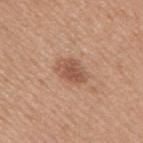site — the right upper arm | image-analysis metrics — a lesion area of about 7 mm², an outline eccentricity of about 0.75 (0 = round, 1 = elongated), and two-axis asymmetry of about 0.2; a border-irregularity index near 2/10, internal color variation of about 2.5 on a 0–10 scale, and peripheral color asymmetry of about 1 | acquisition — ~15 mm crop, total-body skin-cancer survey | lighting — white-light | patient — male, in their mid-50s.